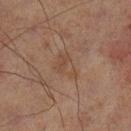follow-up=total-body-photography surveillance lesion; no biopsy
acquisition=~15 mm crop, total-body skin-cancer survey
site=the left lower leg
lighting=cross-polarized
automated metrics=a border-irregularity rating of about 4.5/10 and a peripheral color-asymmetry measure near 1
subject=male, roughly 70 years of age